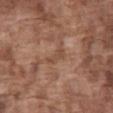Notes:
– workup — imaged on a skin check; not biopsied
– size — ~2.5 mm (longest diameter)
– subject — male, roughly 75 years of age
– tile lighting — white-light illumination
– location — the abdomen
– acquisition — 15 mm crop, total-body photography
– automated metrics — a nevus-likeness score of about 0/100 and a lesion-detection confidence of about 55/100